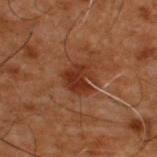{
  "biopsy_status": "not biopsied; imaged during a skin examination",
  "site": "back",
  "image": {
    "source": "total-body photography crop",
    "field_of_view_mm": 15
  },
  "patient": {
    "sex": "male",
    "age_approx": 50
  }
}